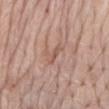notes=imaged on a skin check; not biopsied | image source=15 mm crop, total-body photography | tile lighting=white-light | patient=female, aged 73–77 | anatomic site=the mid back | lesion size=~3 mm (longest diameter).